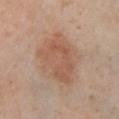• workup · catalogued during a skin exam; not biopsied
• lesion size · about 6.5 mm
• anatomic site · the left lower leg
• acquisition · 15 mm crop, total-body photography
• lighting · cross-polarized
• subject · female, aged 53–57